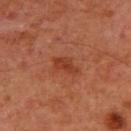Part of a total-body skin-imaging series; this lesion was reviewed on a skin check and was not flagged for biopsy. Measured at roughly 3.5 mm in maximum diameter. The lesion is on the chest. The tile uses cross-polarized illumination. The patient is a male approximately 60 years of age. A close-up tile cropped from a whole-body skin photograph, about 15 mm across.